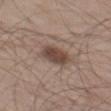Clinical impression:
The lesion was tiled from a total-body skin photograph and was not biopsied.
Context:
The lesion-visualizer software estimated a mean CIELAB color near L≈45 a*≈16 b*≈23 and a normalized lesion–skin contrast near 9. The analysis additionally found a border-irregularity rating of about 2/10, a within-lesion color-variation index near 3.5/10, and peripheral color asymmetry of about 1. The software also gave an automated nevus-likeness rating near 90 out of 100 and a detector confidence of about 100 out of 100 that the crop contains a lesion. A close-up tile cropped from a whole-body skin photograph, about 15 mm across. The subject is a male aged 58–62. The lesion's longest dimension is about 4.5 mm. The tile uses white-light illumination. The lesion is located on the left thigh.